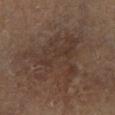The lesion was photographed on a routine skin check and not biopsied; there is no pathology result. Measured at roughly 7 mm in maximum diameter. The patient is a male roughly 65 years of age. The tile uses cross-polarized illumination. The lesion is on the right lower leg. A 15 mm crop from a total-body photograph taken for skin-cancer surveillance.